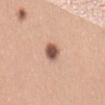workup: catalogued during a skin exam; not biopsied
image source: ~15 mm tile from a whole-body skin photo
illumination: white-light
patient: female, aged around 30
automated lesion analysis: a footprint of about 4.5 mm², an outline eccentricity of about 0.65 (0 = round, 1 = elongated), and a symmetry-axis asymmetry near 0.15; an automated nevus-likeness rating near 100 out of 100
site: the mid back
diameter: about 3 mm